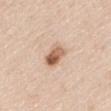Case summary:
• biopsy status: total-body-photography surveillance lesion; no biopsy
• patient: male, about 45 years old
• size: ≈3 mm
• site: the arm
• imaging modality: ~15 mm tile from a whole-body skin photo
• automated metrics: a footprint of about 6 mm², an outline eccentricity of about 0.75 (0 = round, 1 = elongated), and a shape-asymmetry score of about 0.2 (0 = symmetric); a border-irregularity index near 2/10, internal color variation of about 8 on a 0–10 scale, and radial color variation of about 4; a nevus-likeness score of about 95/100 and a detector confidence of about 100 out of 100 that the crop contains a lesion
• lighting: white-light